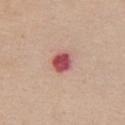follow-up: no biopsy performed (imaged during a skin exam)
diameter: ≈3 mm
illumination: white-light
location: the chest
image: ~15 mm tile from a whole-body skin photo
subject: female, approximately 45 years of age